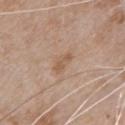biopsy status — catalogued during a skin exam; not biopsied
acquisition — total-body-photography crop, ~15 mm field of view
body site — the front of the torso
subject — male, about 80 years old